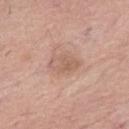Q: Was this lesion biopsied?
A: total-body-photography surveillance lesion; no biopsy
Q: How was this image acquired?
A: total-body-photography crop, ~15 mm field of view
Q: What are the patient's age and sex?
A: female, aged 48–52
Q: Illumination type?
A: white-light
Q: What is the lesion's diameter?
A: ≈4 mm
Q: Where on the body is the lesion?
A: the right thigh
Q: What did automated image analysis measure?
A: an automated nevus-likeness rating near 0 out of 100 and a detector confidence of about 100 out of 100 that the crop contains a lesion